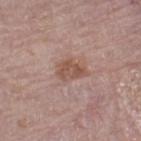The lesion was tiled from a total-body skin photograph and was not biopsied. The tile uses white-light illumination. A male subject aged around 75. Longest diameter approximately 3.5 mm. A 15 mm crop from a total-body photograph taken for skin-cancer surveillance. Located on the left thigh.